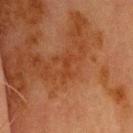Assessment: No biopsy was performed on this lesion — it was imaged during a full skin examination and was not determined to be concerning. Context: Approximately 3 mm at its widest. This is a cross-polarized tile. Automated tile analysis of the lesion measured a mean CIELAB color near L≈34 a*≈24 b*≈33, about 4 CIELAB-L* units darker than the surrounding skin, and a normalized lesion–skin contrast near 4.5. And it measured a color-variation rating of about 0/10 and radial color variation of about 0. The patient is a male about 70 years old. The lesion is located on the head or neck. A 15 mm close-up extracted from a 3D total-body photography capture.